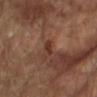workup=total-body-photography surveillance lesion; no biopsy
location=the right upper arm
lesion size=~2.5 mm (longest diameter)
subject=male, approximately 65 years of age
image source=~15 mm crop, total-body skin-cancer survey